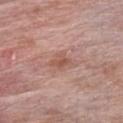Impression: No biopsy was performed on this lesion — it was imaged during a full skin examination and was not determined to be concerning. Context: A male subject, aged 58 to 62. Captured under white-light illumination. The recorded lesion diameter is about 2.5 mm. The lesion is located on the chest. A 15 mm close-up tile from a total-body photography series done for melanoma screening. The total-body-photography lesion software estimated a lesion area of about 3.5 mm² and an eccentricity of roughly 0.75. The software also gave a nevus-likeness score of about 0/100 and a detector confidence of about 100 out of 100 that the crop contains a lesion.